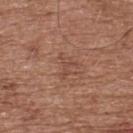  biopsy_status: not biopsied; imaged during a skin examination
  image:
    source: total-body photography crop
    field_of_view_mm: 15
  patient:
    sex: male
    age_approx: 75
  site: back